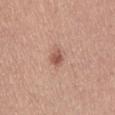Part of a total-body skin-imaging series; this lesion was reviewed on a skin check and was not flagged for biopsy.
The lesion is located on the back.
This is a white-light tile.
A region of skin cropped from a whole-body photographic capture, roughly 15 mm wide.
The lesion's longest dimension is about 2.5 mm.
A female subject, approximately 45 years of age.